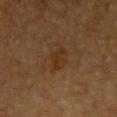Part of a total-body skin-imaging series; this lesion was reviewed on a skin check and was not flagged for biopsy.
An algorithmic analysis of the crop reported a nevus-likeness score of about 5/100.
From the chest.
Imaged with cross-polarized lighting.
A female patient, aged approximately 55.
A 15 mm crop from a total-body photograph taken for skin-cancer surveillance.
The lesion's longest dimension is about 3 mm.